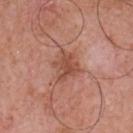Case summary:
• notes: total-body-photography surveillance lesion; no biopsy
• image: total-body-photography crop, ~15 mm field of view
• body site: the chest
• subject: male, aged 68 to 72